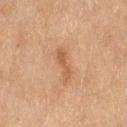notes: total-body-photography surveillance lesion; no biopsy
imaging modality: total-body-photography crop, ~15 mm field of view
location: the right thigh
automated lesion analysis: an outline eccentricity of about 0.95 (0 = round, 1 = elongated) and a shape-asymmetry score of about 0.4 (0 = symmetric); an average lesion color of about L≈49 a*≈20 b*≈31 (CIELAB), about 8 CIELAB-L* units darker than the surrounding skin, and a normalized border contrast of about 6; a classifier nevus-likeness of about 5/100 and lesion-presence confidence of about 100/100
size: ~4 mm (longest diameter)
illumination: cross-polarized illumination
patient: female, roughly 80 years of age